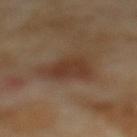Q: Is there a histopathology result?
A: total-body-photography surveillance lesion; no biopsy
Q: Who is the patient?
A: female, about 55 years old
Q: What kind of image is this?
A: total-body-photography crop, ~15 mm field of view
Q: Lesion location?
A: the mid back